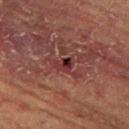follow-up: catalogued during a skin exam; not biopsied
acquisition: total-body-photography crop, ~15 mm field of view
anatomic site: the leg
TBP lesion metrics: an area of roughly 4 mm², an eccentricity of roughly 0.75, and a shape-asymmetry score of about 0.4 (0 = symmetric)
lighting: cross-polarized
subject: male, about 65 years old
lesion diameter: ≈3 mm